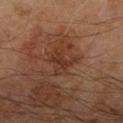The lesion was photographed on a routine skin check and not biopsied; there is no pathology result. A roughly 15 mm field-of-view crop from a total-body skin photograph. A male subject, about 65 years old. On the right forearm.